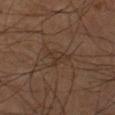{"biopsy_status": "not biopsied; imaged during a skin examination", "lighting": "cross-polarized", "lesion_size": {"long_diameter_mm_approx": 3.5}, "image": {"source": "total-body photography crop", "field_of_view_mm": 15}, "site": "leg", "patient": {"sex": "male", "age_approx": 60}}